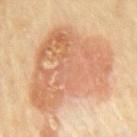Image and clinical context:
Automated tile analysis of the lesion measured a border-irregularity rating of about 4/10, a within-lesion color-variation index near 5.5/10, and peripheral color asymmetry of about 2. The tile uses cross-polarized illumination. A 15 mm crop from a total-body photograph taken for skin-cancer surveillance. The lesion is located on the back. The subject is a male aged around 70.